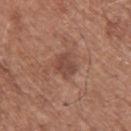biopsy_status: not biopsied; imaged during a skin examination
lighting: white-light
site: upper back
lesion_size:
  long_diameter_mm_approx: 3.0
patient:
  sex: male
  age_approx: 75
image:
  source: total-body photography crop
  field_of_view_mm: 15